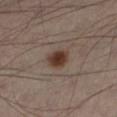The lesion was tiled from a total-body skin photograph and was not biopsied.
The tile uses cross-polarized illumination.
The lesion-visualizer software estimated about 12 CIELAB-L* units darker than the surrounding skin. The software also gave an automated nevus-likeness rating near 100 out of 100.
The subject is a male aged around 50.
From the left leg.
A 15 mm crop from a total-body photograph taken for skin-cancer surveillance.